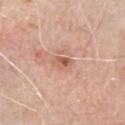Recorded during total-body skin imaging; not selected for excision or biopsy. A 15 mm crop from a total-body photograph taken for skin-cancer surveillance. The patient is a male aged approximately 80. This is a white-light tile. Measured at roughly 2.5 mm in maximum diameter. The lesion is on the chest.